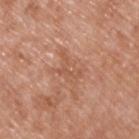Case summary:
* notes · imaged on a skin check; not biopsied
* size · ≈4 mm
* subject · male, aged 48 to 52
* image source · ~15 mm tile from a whole-body skin photo
* anatomic site · the upper back
* tile lighting · white-light